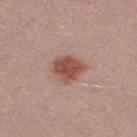Captured during whole-body skin photography for melanoma surveillance; the lesion was not biopsied. The lesion is located on the right thigh. Measured at roughly 3.5 mm in maximum diameter. Automated image analysis of the tile measured a footprint of about 8.5 mm², an outline eccentricity of about 0.6 (0 = round, 1 = elongated), and a shape-asymmetry score of about 0.25 (0 = symmetric). And it measured an average lesion color of about L≈50 a*≈24 b*≈26 (CIELAB), roughly 13 lightness units darker than nearby skin, and a lesion-to-skin contrast of about 9 (normalized; higher = more distinct). It also reported a border-irregularity index near 2/10, a within-lesion color-variation index near 3.5/10, and peripheral color asymmetry of about 1. A 15 mm crop from a total-body photograph taken for skin-cancer surveillance. This is a white-light tile. A female patient, in their mid-20s.